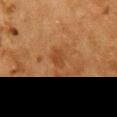| key | value |
|---|---|
| workup | total-body-photography surveillance lesion; no biopsy |
| patient | female, aged 48 to 52 |
| anatomic site | the chest |
| imaging modality | total-body-photography crop, ~15 mm field of view |